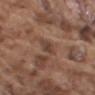Imaged during a routine full-body skin examination; the lesion was not biopsied and no histopathology is available. The subject is a male in their mid- to late 70s. Located on the right upper arm. A close-up tile cropped from a whole-body skin photograph, about 15 mm across.